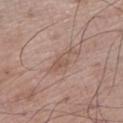workup — no biopsy performed (imaged during a skin exam)
size — ≈3.5 mm
tile lighting — white-light illumination
anatomic site — the left lower leg
subject — male, aged 68 to 72
automated lesion analysis — a lesion color around L≈54 a*≈18 b*≈26 in CIELAB, roughly 7 lightness units darker than nearby skin, and a lesion-to-skin contrast of about 5.5 (normalized; higher = more distinct); border irregularity of about 4 on a 0–10 scale and radial color variation of about 1
image source — 15 mm crop, total-body photography